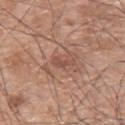Clinical impression:
Part of a total-body skin-imaging series; this lesion was reviewed on a skin check and was not flagged for biopsy.
Context:
The lesion's longest dimension is about 3 mm. The patient is a male in their mid-60s. Imaged with white-light lighting. The lesion-visualizer software estimated an average lesion color of about L≈50 a*≈21 b*≈28 (CIELAB), a lesion–skin lightness drop of about 8, and a lesion-to-skin contrast of about 5.5 (normalized; higher = more distinct). The software also gave internal color variation of about 0 on a 0–10 scale and a peripheral color-asymmetry measure near 0. And it measured a classifier nevus-likeness of about 0/100. The lesion is on the back. A lesion tile, about 15 mm wide, cut from a 3D total-body photograph.